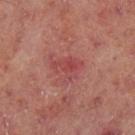Captured during whole-body skin photography for melanoma surveillance; the lesion was not biopsied. Cropped from a whole-body photographic skin survey; the tile spans about 15 mm. Longest diameter approximately 3.5 mm. From the left lower leg. A male subject aged approximately 70.